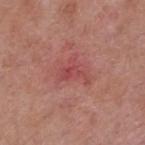Case summary:
– notes: imaged on a skin check; not biopsied
– patient: female, aged 58 to 62
– location: the upper back
– lighting: white-light
– TBP lesion metrics: roughly 7 lightness units darker than nearby skin and a normalized border contrast of about 5.5; a classifier nevus-likeness of about 0/100 and a detector confidence of about 100 out of 100 that the crop contains a lesion
– imaging modality: ~15 mm crop, total-body skin-cancer survey